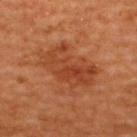Part of a total-body skin-imaging series; this lesion was reviewed on a skin check and was not flagged for biopsy.
Longest diameter approximately 7 mm.
A lesion tile, about 15 mm wide, cut from a 3D total-body photograph.
Imaged with cross-polarized lighting.
The lesion is on the back.
A female patient, in their mid- to late 50s.